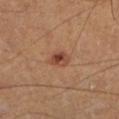Part of a total-body skin-imaging series; this lesion was reviewed on a skin check and was not flagged for biopsy. This is a cross-polarized tile. About 2.5 mm across. A 15 mm close-up extracted from a 3D total-body photography capture. A male patient aged around 65. The lesion-visualizer software estimated an outline eccentricity of about 0.65 (0 = round, 1 = elongated) and a shape-asymmetry score of about 0.25 (0 = symmetric). It also reported a mean CIELAB color near L≈44 a*≈24 b*≈31 and a lesion-to-skin contrast of about 8.5 (normalized; higher = more distinct). The analysis additionally found a border-irregularity rating of about 2/10, a color-variation rating of about 6.5/10, and a peripheral color-asymmetry measure near 2.5. Located on the left lower leg.